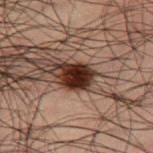| field | value |
|---|---|
| biopsy status | total-body-photography surveillance lesion; no biopsy |
| anatomic site | the right thigh |
| TBP lesion metrics | a border-irregularity index near 2.5/10 and peripheral color asymmetry of about 1; an automated nevus-likeness rating near 100 out of 100 and lesion-presence confidence of about 100/100 |
| diameter | ≈3.5 mm |
| patient | male, aged 48 to 52 |
| illumination | cross-polarized illumination |
| image | ~15 mm crop, total-body skin-cancer survey |